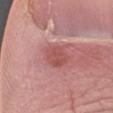- workup — total-body-photography surveillance lesion; no biopsy